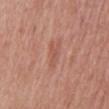Recorded during total-body skin imaging; not selected for excision or biopsy. On the mid back. A male patient, aged 63 to 67. This image is a 15 mm lesion crop taken from a total-body photograph.